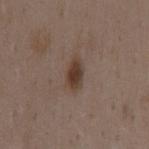follow-up: catalogued during a skin exam; not biopsied.